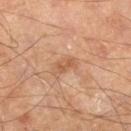Case summary:
- follow-up — total-body-photography surveillance lesion; no biopsy
- illumination — cross-polarized
- site — the right lower leg
- image-analysis metrics — a lesion area of about 3.5 mm² and a shape eccentricity near 0.85; border irregularity of about 3 on a 0–10 scale and a within-lesion color-variation index near 1/10
- acquisition — ~15 mm crop, total-body skin-cancer survey
- patient — male, roughly 55 years of age